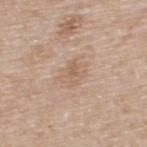Imaged during a routine full-body skin examination; the lesion was not biopsied and no histopathology is available. The tile uses white-light illumination. A roughly 15 mm field-of-view crop from a total-body skin photograph. Located on the upper back. Automated tile analysis of the lesion measured an area of roughly 3.5 mm², an outline eccentricity of about 0.85 (0 = round, 1 = elongated), and a shape-asymmetry score of about 0.25 (0 = symmetric). It also reported a border-irregularity index near 2.5/10, a color-variation rating of about 2/10, and peripheral color asymmetry of about 0.5. It also reported a nevus-likeness score of about 0/100 and a detector confidence of about 100 out of 100 that the crop contains a lesion. A male patient aged approximately 65.